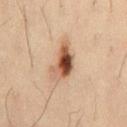A 15 mm crop from a total-body photograph taken for skin-cancer surveillance. From the mid back. The subject is a male roughly 35 years of age.